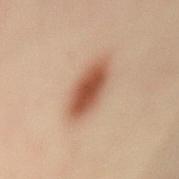follow-up: no biopsy performed (imaged during a skin exam)
location: the back
patient: female, aged 38–42
acquisition: total-body-photography crop, ~15 mm field of view